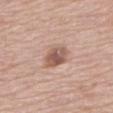| field | value |
|---|---|
| notes | imaged on a skin check; not biopsied |
| diameter | ≈4 mm |
| imaging modality | ~15 mm tile from a whole-body skin photo |
| illumination | white-light |
| automated lesion analysis | a lesion color around L≈55 a*≈20 b*≈26 in CIELAB, a lesion–skin lightness drop of about 13, and a normalized border contrast of about 8.5 |
| patient | male, aged 83 or over |
| body site | the upper back |